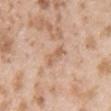Part of a total-body skin-imaging series; this lesion was reviewed on a skin check and was not flagged for biopsy. Imaged with white-light lighting. Longest diameter approximately 3 mm. On the right upper arm. A male patient, roughly 25 years of age. A 15 mm close-up extracted from a 3D total-body photography capture. Automated tile analysis of the lesion measured an average lesion color of about L≈61 a*≈20 b*≈32 (CIELAB), roughly 9 lightness units darker than nearby skin, and a lesion-to-skin contrast of about 6 (normalized; higher = more distinct).